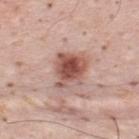Part of a total-body skin-imaging series; this lesion was reviewed on a skin check and was not flagged for biopsy.
A 15 mm crop from a total-body photograph taken for skin-cancer surveillance.
Captured under white-light illumination.
A male subject, about 35 years old.
The lesion is on the mid back.
The total-body-photography lesion software estimated an outline eccentricity of about 0.5 (0 = round, 1 = elongated) and a symmetry-axis asymmetry near 0.3. The software also gave a mean CIELAB color near L≈53 a*≈24 b*≈26 and roughly 15 lightness units darker than nearby skin. It also reported a border-irregularity rating of about 3.5/10 and radial color variation of about 1.5. The analysis additionally found a classifier nevus-likeness of about 90/100 and a lesion-detection confidence of about 100/100.
Measured at roughly 4 mm in maximum diameter.